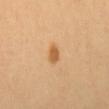<record>
<image>
  <source>total-body photography crop</source>
  <field_of_view_mm>15</field_of_view_mm>
</image>
<automated_metrics>
  <border_irregularity_0_10>2.0</border_irregularity_0_10>
  <color_variation_0_10>2.0</color_variation_0_10>
  <peripheral_color_asymmetry>0.5</peripheral_color_asymmetry>
  <lesion_detection_confidence_0_100>100</lesion_detection_confidence_0_100>
</automated_metrics>
<site>head or neck</site>
<patient>
  <sex>female</sex>
  <age_approx>40</age_approx>
</patient>
</record>